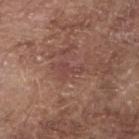workup — catalogued during a skin exam; not biopsied
anatomic site — the right forearm
image source — ~15 mm crop, total-body skin-cancer survey
patient — male, approximately 75 years of age
tile lighting — white-light illumination
lesion diameter — ≈2.5 mm